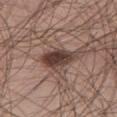The lesion was tiled from a total-body skin photograph and was not biopsied.
A male patient, roughly 55 years of age.
This image is a 15 mm lesion crop taken from a total-body photograph.
The lesion is located on the right thigh.
Automated tile analysis of the lesion measured an average lesion color of about L≈40 a*≈17 b*≈21 (CIELAB) and a lesion-to-skin contrast of about 11 (normalized; higher = more distinct). The analysis additionally found border irregularity of about 3.5 on a 0–10 scale, a color-variation rating of about 5.5/10, and peripheral color asymmetry of about 2. The analysis additionally found lesion-presence confidence of about 100/100.
The lesion's longest dimension is about 4.5 mm.